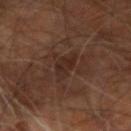Case summary:
- lesion size: ≈4 mm
- patient: male, aged approximately 60
- image: ~15 mm crop, total-body skin-cancer survey
- tile lighting: cross-polarized
- TBP lesion metrics: a footprint of about 5.5 mm²; border irregularity of about 3.5 on a 0–10 scale, internal color variation of about 2.5 on a 0–10 scale, and radial color variation of about 1; a nevus-likeness score of about 5/100 and lesion-presence confidence of about 95/100
- body site: the right arm